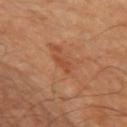notes: catalogued during a skin exam; not biopsied | image source: total-body-photography crop, ~15 mm field of view | site: the left upper arm | lesion size: about 2.5 mm | illumination: cross-polarized illumination | subject: male, aged around 70.